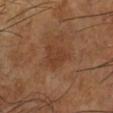Clinical impression: Captured during whole-body skin photography for melanoma surveillance; the lesion was not biopsied. Acquisition and patient details: The lesion is located on the leg. A male patient roughly 65 years of age. A 15 mm crop from a total-body photograph taken for skin-cancer surveillance.